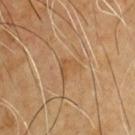Imaged during a routine full-body skin examination; the lesion was not biopsied and no histopathology is available. The lesion is on the chest. The total-body-photography lesion software estimated a footprint of about 3.5 mm², a shape eccentricity near 0.65, and two-axis asymmetry of about 0.6. The analysis additionally found a lesion–skin lightness drop of about 6. And it measured a border-irregularity rating of about 6/10 and internal color variation of about 1 on a 0–10 scale. The analysis additionally found a nevus-likeness score of about 0/100. The patient is a male aged approximately 65. A region of skin cropped from a whole-body photographic capture, roughly 15 mm wide. This is a cross-polarized tile.